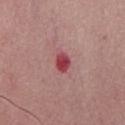Notes:
- lighting — white-light
- location — the chest
- automated metrics — a lesion color around L≈45 a*≈36 b*≈21 in CIELAB, a lesion–skin lightness drop of about 14, and a normalized border contrast of about 10; a border-irregularity index near 2/10, internal color variation of about 3 on a 0–10 scale, and radial color variation of about 1
- size — ≈2.5 mm
- patient — male, in their mid-50s
- image source — total-body-photography crop, ~15 mm field of view